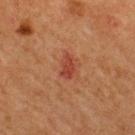{
  "site": "back",
  "lesion_size": {
    "long_diameter_mm_approx": 2.5
  },
  "image": {
    "source": "total-body photography crop",
    "field_of_view_mm": 15
  },
  "patient": {
    "sex": "male",
    "age_approx": 65
  }
}